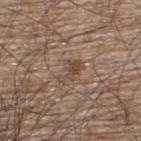biopsy status: no biopsy performed (imaged during a skin exam)
patient: male, in their mid-60s
anatomic site: the upper back
acquisition: total-body-photography crop, ~15 mm field of view
size: ~3 mm (longest diameter)
TBP lesion metrics: an area of roughly 4.5 mm², an eccentricity of roughly 0.8, and two-axis asymmetry of about 0.45; a lesion color around L≈46 a*≈16 b*≈25 in CIELAB and a lesion-to-skin contrast of about 6.5 (normalized; higher = more distinct); peripheral color asymmetry of about 0.5; a classifier nevus-likeness of about 0/100 and a detector confidence of about 95 out of 100 that the crop contains a lesion